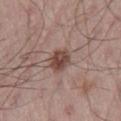The lesion was photographed on a routine skin check and not biopsied; there is no pathology result. A male patient aged around 55. On the right thigh. A close-up tile cropped from a whole-body skin photograph, about 15 mm across.